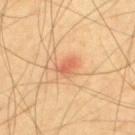Q: Was this lesion biopsied?
A: imaged on a skin check; not biopsied
Q: How was this image acquired?
A: total-body-photography crop, ~15 mm field of view
Q: Where on the body is the lesion?
A: the upper back
Q: What are the patient's age and sex?
A: male, aged approximately 65